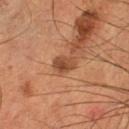imaging modality — 15 mm crop, total-body photography
subject — male, aged approximately 55
location — the left lower leg
diameter — ≈2.5 mm
tile lighting — cross-polarized illumination